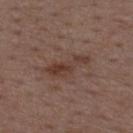follow-up — no biopsy performed (imaged during a skin exam)
acquisition — ~15 mm tile from a whole-body skin photo
lesion diameter — ~5.5 mm (longest diameter)
illumination — white-light illumination
subject — male, in their 50s
automated lesion analysis — an automated nevus-likeness rating near 15 out of 100
anatomic site — the mid back